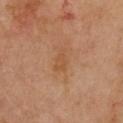Image and clinical context: The lesion is located on the chest. A female subject aged approximately 50. A region of skin cropped from a whole-body photographic capture, roughly 15 mm wide.